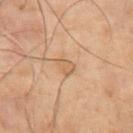size — ~2.5 mm (longest diameter)
location — the chest
patient — male, aged 68 to 72
image source — 15 mm crop, total-body photography